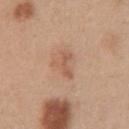Automated tile analysis of the lesion measured a footprint of about 5.5 mm² and a symmetry-axis asymmetry near 0.55. It also reported a lesion–skin lightness drop of about 8 and a normalized border contrast of about 5.5. And it measured a border-irregularity rating of about 6/10 and peripheral color asymmetry of about 1. On the chest. The recorded lesion diameter is about 3.5 mm. A female patient, aged around 30. Captured under white-light illumination. This image is a 15 mm lesion crop taken from a total-body photograph.